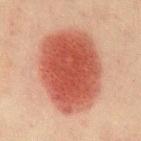Clinical impression: Captured during whole-body skin photography for melanoma surveillance; the lesion was not biopsied. Context: Cropped from a whole-body photographic skin survey; the tile spans about 15 mm. The subject is a female aged 48 to 52. On the chest.